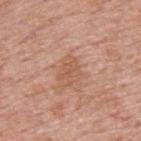workup: total-body-photography surveillance lesion; no biopsy | image-analysis metrics: a footprint of about 3.5 mm², an outline eccentricity of about 0.75 (0 = round, 1 = elongated), and a shape-asymmetry score of about 0.45 (0 = symmetric); an average lesion color of about L≈56 a*≈23 b*≈31 (CIELAB), roughly 7 lightness units darker than nearby skin, and a lesion-to-skin contrast of about 5 (normalized; higher = more distinct) | imaging modality: ~15 mm tile from a whole-body skin photo | lesion diameter: about 3 mm | patient: male, approximately 75 years of age | site: the upper back | tile lighting: white-light illumination.